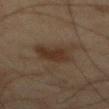Clinical summary: From the abdomen. A roughly 15 mm field-of-view crop from a total-body skin photograph. The lesion's longest dimension is about 4 mm. This is a cross-polarized tile. The patient is a male aged 63–67. The lesion-visualizer software estimated an outline eccentricity of about 0.45 (0 = round, 1 = elongated) and a symmetry-axis asymmetry near 0.2.